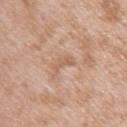Clinical impression:
Captured during whole-body skin photography for melanoma surveillance; the lesion was not biopsied.
Image and clinical context:
A male subject aged approximately 50. From the left upper arm. A roughly 15 mm field-of-view crop from a total-body skin photograph. Longest diameter approximately 3 mm. The tile uses white-light illumination.